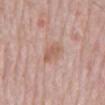No biopsy was performed on this lesion — it was imaged during a full skin examination and was not determined to be concerning. A lesion tile, about 15 mm wide, cut from a 3D total-body photograph. A male patient, roughly 80 years of age. The lesion is on the mid back.